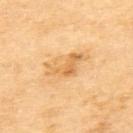Q: Was this lesion biopsied?
A: total-body-photography surveillance lesion; no biopsy
Q: What is the lesion's diameter?
A: ≈5 mm
Q: What kind of image is this?
A: total-body-photography crop, ~15 mm field of view
Q: Who is the patient?
A: male, aged 68–72
Q: Automated lesion metrics?
A: a lesion area of about 8.5 mm², an outline eccentricity of about 0.85 (0 = round, 1 = elongated), and a symmetry-axis asymmetry near 0.55; an average lesion color of about L≈64 a*≈19 b*≈44 (CIELAB), roughly 9 lightness units darker than nearby skin, and a normalized lesion–skin contrast near 6.5
Q: How was the tile lit?
A: cross-polarized
Q: Lesion location?
A: the upper back